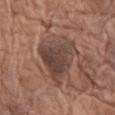  biopsy_status: not biopsied; imaged during a skin examination
  site: left thigh
  patient:
    sex: female
    age_approx: 75
  lesion_size:
    long_diameter_mm_approx: 6.0
  image:
    source: total-body photography crop
    field_of_view_mm: 15